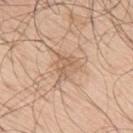The subject is a male in their 70s.
From the upper back.
Cropped from a whole-body photographic skin survey; the tile spans about 15 mm.